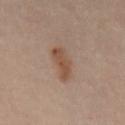Impression: Imaged during a routine full-body skin examination; the lesion was not biopsied and no histopathology is available. Acquisition and patient details: Longest diameter approximately 4.5 mm. A male subject, roughly 50 years of age. Captured under cross-polarized illumination. This image is a 15 mm lesion crop taken from a total-body photograph. The lesion is located on the mid back.